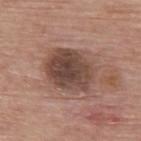Q: Was a biopsy performed?
A: catalogued during a skin exam; not biopsied
Q: Who is the patient?
A: male, about 85 years old or older
Q: Lesion size?
A: ~5.5 mm (longest diameter)
Q: Illumination type?
A: white-light
Q: What is the imaging modality?
A: 15 mm crop, total-body photography
Q: Lesion location?
A: the upper back